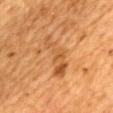No biopsy was performed on this lesion — it was imaged during a full skin examination and was not determined to be concerning.
A female patient approximately 55 years of age.
Cropped from a total-body skin-imaging series; the visible field is about 15 mm.
On the back.